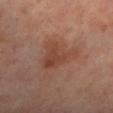<case>
  <biopsy_status>not biopsied; imaged during a skin examination</biopsy_status>
  <site>leg</site>
  <image>
    <source>total-body photography crop</source>
    <field_of_view_mm>15</field_of_view_mm>
  </image>
  <lesion_size>
    <long_diameter_mm_approx>5.0</long_diameter_mm_approx>
  </lesion_size>
  <patient>
    <sex>female</sex>
    <age_approx>60</age_approx>
  </patient>
</case>